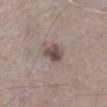Assessment: The lesion was tiled from a total-body skin photograph and was not biopsied. Clinical summary: A male subject, in their mid-70s. The lesion is on the right lower leg. A lesion tile, about 15 mm wide, cut from a 3D total-body photograph. Automated tile analysis of the lesion measured a footprint of about 5 mm², a shape eccentricity near 0.6, and a shape-asymmetry score of about 0.25 (0 = symmetric). The software also gave border irregularity of about 2.5 on a 0–10 scale, internal color variation of about 4 on a 0–10 scale, and radial color variation of about 1.5. About 3 mm across. Imaged with white-light lighting.